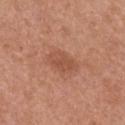| key | value |
|---|---|
| biopsy status | imaged on a skin check; not biopsied |
| image | ~15 mm tile from a whole-body skin photo |
| anatomic site | the front of the torso |
| lighting | white-light |
| patient | female, aged around 50 |
| size | ~4 mm (longest diameter) |